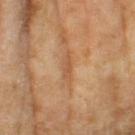Assessment:
Imaged during a routine full-body skin examination; the lesion was not biopsied and no histopathology is available.
Image and clinical context:
The lesion is on the left thigh. A female subject, aged 58 to 62. A 15 mm close-up extracted from a 3D total-body photography capture.